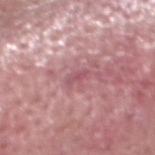<lesion>
<biopsy_status>not biopsied; imaged during a skin examination</biopsy_status>
<patient>
  <sex>male</sex>
  <age_approx>50</age_approx>
</patient>
<automated_metrics>
  <area_mm2_approx>2.5</area_mm2_approx>
  <eccentricity>0.9</eccentricity>
  <shape_asymmetry>0.4</shape_asymmetry>
  <lesion_detection_confidence_0_100>55</lesion_detection_confidence_0_100>
</automated_metrics>
<lighting>white-light</lighting>
<site>head or neck</site>
<image>
  <source>total-body photography crop</source>
  <field_of_view_mm>15</field_of_view_mm>
</image>
<lesion_size>
  <long_diameter_mm_approx>2.5</long_diameter_mm_approx>
</lesion_size>
</lesion>